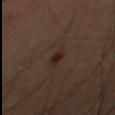Impression:
Part of a total-body skin-imaging series; this lesion was reviewed on a skin check and was not flagged for biopsy.
Background:
The tile uses cross-polarized illumination. The subject is a male approximately 60 years of age. The lesion is on the right thigh. An algorithmic analysis of the crop reported a lesion area of about 3 mm² and a shape eccentricity near 0.85. It also reported a lesion color around L≈16 a*≈13 b*≈14 in CIELAB, about 6 CIELAB-L* units darker than the surrounding skin, and a normalized lesion–skin contrast near 8.5. It also reported a border-irregularity index near 3/10 and peripheral color asymmetry of about 0.5. A roughly 15 mm field-of-view crop from a total-body skin photograph.